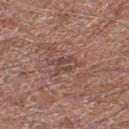Imaged during a routine full-body skin examination; the lesion was not biopsied and no histopathology is available.
The recorded lesion diameter is about 3 mm.
Cropped from a total-body skin-imaging series; the visible field is about 15 mm.
The lesion is on the right lower leg.
A male patient in their 80s.
Imaged with white-light lighting.